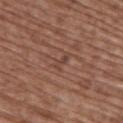No biopsy was performed on this lesion — it was imaged during a full skin examination and was not determined to be concerning.
The lesion is on the upper back.
The subject is a female roughly 75 years of age.
The tile uses white-light illumination.
A lesion tile, about 15 mm wide, cut from a 3D total-body photograph.
Longest diameter approximately 2.5 mm.